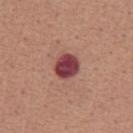<record>
<lighting>white-light</lighting>
<image>
  <source>total-body photography crop</source>
  <field_of_view_mm>15</field_of_view_mm>
</image>
<site>abdomen</site>
<patient>
  <sex>male</sex>
  <age_approx>45</age_approx>
</patient>
</record>